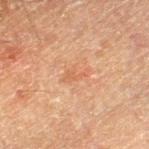Part of a total-body skin-imaging series; this lesion was reviewed on a skin check and was not flagged for biopsy.
A lesion tile, about 15 mm wide, cut from a 3D total-body photograph.
The tile uses cross-polarized illumination.
Longest diameter approximately 3 mm.
A male subject aged around 60.
The lesion is on the left thigh.